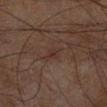Clinical impression: The lesion was photographed on a routine skin check and not biopsied; there is no pathology result. Background: From the right lower leg. The tile uses cross-polarized illumination. A male patient, in their 60s. Longest diameter approximately 2.5 mm. A lesion tile, about 15 mm wide, cut from a 3D total-body photograph.